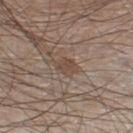Impression: Recorded during total-body skin imaging; not selected for excision or biopsy. Context: This is a white-light tile. Longest diameter approximately 2.5 mm. A male subject in their 60s. The total-body-photography lesion software estimated a lesion area of about 4 mm², a shape eccentricity near 0.6, and two-axis asymmetry of about 0.3. The software also gave a classifier nevus-likeness of about 5/100 and lesion-presence confidence of about 95/100. Located on the right lower leg. A roughly 15 mm field-of-view crop from a total-body skin photograph.